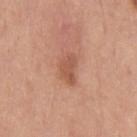<record>
  <biopsy_status>not biopsied; imaged during a skin examination</biopsy_status>
  <lesion_size>
    <long_diameter_mm_approx>3.5</long_diameter_mm_approx>
  </lesion_size>
  <lighting>white-light</lighting>
  <patient>
    <sex>male</sex>
    <age_approx>40</age_approx>
  </patient>
  <site>back</site>
  <image>
    <source>total-body photography crop</source>
    <field_of_view_mm>15</field_of_view_mm>
  </image>
</record>